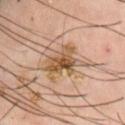workup — catalogued during a skin exam; not biopsied
illumination — cross-polarized
diameter — ≈5 mm
patient — male, approximately 50 years of age
imaging modality — ~15 mm tile from a whole-body skin photo
site — the abdomen
automated metrics — a lesion area of about 11 mm², a shape eccentricity near 0.7, and a shape-asymmetry score of about 0.4 (0 = symmetric); a lesion color around L≈54 a*≈18 b*≈34 in CIELAB and a lesion–skin lightness drop of about 12; a border-irregularity rating of about 6/10, a within-lesion color-variation index near 6/10, and a peripheral color-asymmetry measure near 2; a classifier nevus-likeness of about 60/100